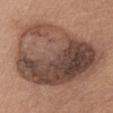<case>
  <biopsy_status>not biopsied; imaged during a skin examination</biopsy_status>
  <site>arm</site>
  <image>
    <source>total-body photography crop</source>
    <field_of_view_mm>15</field_of_view_mm>
  </image>
  <lesion_size>
    <long_diameter_mm_approx>12.0</long_diameter_mm_approx>
  </lesion_size>
  <lighting>white-light</lighting>
  <patient>
    <sex>male</sex>
    <age_approx>60</age_approx>
  </patient>
</case>